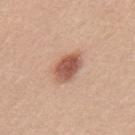  biopsy_status: not biopsied; imaged during a skin examination
  patient:
    sex: female
    age_approx: 35
  site: mid back
  image:
    source: total-body photography crop
    field_of_view_mm: 15